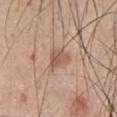The lesion was tiled from a total-body skin photograph and was not biopsied. A male patient aged 63 to 67. Cropped from a total-body skin-imaging series; the visible field is about 15 mm. On the chest. The tile uses white-light illumination. An algorithmic analysis of the crop reported a lesion area of about 5.5 mm², an eccentricity of roughly 0.45, and a symmetry-axis asymmetry near 0.4. And it measured a mean CIELAB color near L≈56 a*≈18 b*≈31 and roughly 9 lightness units darker than nearby skin. The software also gave a border-irregularity index near 3.5/10, internal color variation of about 3 on a 0–10 scale, and radial color variation of about 1.